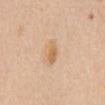Part of a total-body skin-imaging series; this lesion was reviewed on a skin check and was not flagged for biopsy.
From the chest.
A 15 mm close-up tile from a total-body photography series done for melanoma screening.
A female patient, about 70 years old.